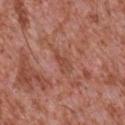{
  "image": {
    "source": "total-body photography crop",
    "field_of_view_mm": 15
  },
  "automated_metrics": {
    "area_mm2_approx": 3.5,
    "eccentricity": 0.9,
    "shape_asymmetry": 0.5,
    "cielab_L": 47,
    "cielab_a": 26,
    "cielab_b": 30,
    "vs_skin_contrast_norm": 5.5,
    "border_irregularity_0_10": 5.5,
    "color_variation_0_10": 1.5,
    "peripheral_color_asymmetry": 0.5,
    "nevus_likeness_0_100": 0,
    "lesion_detection_confidence_0_100": 75
  },
  "lesion_size": {
    "long_diameter_mm_approx": 3.0
  },
  "patient": {
    "sex": "male",
    "age_approx": 45
  },
  "site": "chest"
}